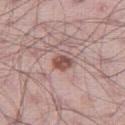Case summary:
- follow-up — catalogued during a skin exam; not biopsied
- tile lighting — white-light illumination
- lesion size — about 2.5 mm
- anatomic site — the leg
- acquisition — ~15 mm crop, total-body skin-cancer survey
- patient — male, aged around 50